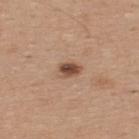<case>
<biopsy_status>not biopsied; imaged during a skin examination</biopsy_status>
<site>upper back</site>
<lighting>white-light</lighting>
<image>
  <source>total-body photography crop</source>
  <field_of_view_mm>15</field_of_view_mm>
</image>
<lesion_size>
  <long_diameter_mm_approx>2.5</long_diameter_mm_approx>
</lesion_size>
<patient>
  <sex>male</sex>
  <age_approx>35</age_approx>
</patient>
</case>